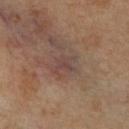Q: Is there a histopathology result?
A: imaged on a skin check; not biopsied
Q: What are the patient's age and sex?
A: female, aged approximately 50
Q: What is the anatomic site?
A: the right lower leg
Q: What kind of image is this?
A: total-body-photography crop, ~15 mm field of view
Q: What did automated image analysis measure?
A: a lesion area of about 5 mm², an eccentricity of roughly 0.75, and two-axis asymmetry of about 0.35; a lesion color around L≈44 a*≈17 b*≈22 in CIELAB, a lesion–skin lightness drop of about 6, and a lesion-to-skin contrast of about 5.5 (normalized; higher = more distinct)
Q: What lighting was used for the tile?
A: cross-polarized illumination